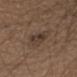Imaged during a routine full-body skin examination; the lesion was not biopsied and no histopathology is available. Automated image analysis of the tile measured a footprint of about 6 mm², an eccentricity of roughly 0.75, and a shape-asymmetry score of about 0.25 (0 = symmetric). A female subject, aged approximately 40. This is a white-light tile. Located on the back. Cropped from a whole-body photographic skin survey; the tile spans about 15 mm.